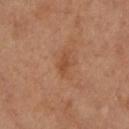Recorded during total-body skin imaging; not selected for excision or biopsy. The lesion's longest dimension is about 2.5 mm. The subject is a female about 65 years old. A lesion tile, about 15 mm wide, cut from a 3D total-body photograph. The lesion is located on the leg. Imaged with cross-polarized lighting.